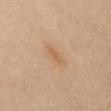The lesion was tiled from a total-body skin photograph and was not biopsied.
The lesion is on the abdomen.
Captured under cross-polarized illumination.
Cropped from a total-body skin-imaging series; the visible field is about 15 mm.
Automated tile analysis of the lesion measured an area of roughly 2.5 mm², an outline eccentricity of about 0.95 (0 = round, 1 = elongated), and a symmetry-axis asymmetry near 0.3. And it measured a lesion–skin lightness drop of about 7 and a normalized lesion–skin contrast near 5.5.
Measured at roughly 3 mm in maximum diameter.
A female patient approximately 40 years of age.